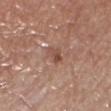Impression:
Part of a total-body skin-imaging series; this lesion was reviewed on a skin check and was not flagged for biopsy.
Context:
Located on the leg. A roughly 15 mm field-of-view crop from a total-body skin photograph. A female subject in their mid- to late 70s.